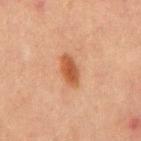workup: no biopsy performed (imaged during a skin exam)
automated metrics: roughly 10 lightness units darker than nearby skin and a normalized lesion–skin contrast near 8.5; border irregularity of about 2 on a 0–10 scale, internal color variation of about 2.5 on a 0–10 scale, and radial color variation of about 1
body site: the chest
lighting: cross-polarized
acquisition: 15 mm crop, total-body photography
size: about 4 mm
patient: male, approximately 65 years of age